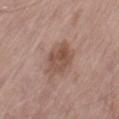Clinical impression: Part of a total-body skin-imaging series; this lesion was reviewed on a skin check and was not flagged for biopsy. Acquisition and patient details: An algorithmic analysis of the crop reported a shape-asymmetry score of about 0.25 (0 = symmetric). The analysis additionally found border irregularity of about 2.5 on a 0–10 scale, a color-variation rating of about 4/10, and a peripheral color-asymmetry measure near 1.5. The software also gave a lesion-detection confidence of about 100/100. This is a white-light tile. A female subject in their mid- to late 70s. This image is a 15 mm lesion crop taken from a total-body photograph. On the right thigh.